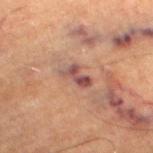Notes:
• follow-up · imaged on a skin check; not biopsied
• body site · the leg
• image · total-body-photography crop, ~15 mm field of view
• subject · male, aged around 85 or older